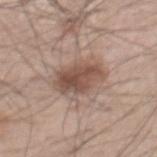Q: Was a biopsy performed?
A: total-body-photography surveillance lesion; no biopsy
Q: Patient demographics?
A: male, aged 48–52
Q: What is the imaging modality?
A: 15 mm crop, total-body photography
Q: What is the anatomic site?
A: the mid back
Q: Lesion size?
A: ~5 mm (longest diameter)
Q: How was the tile lit?
A: white-light illumination